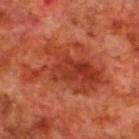workup: total-body-photography surveillance lesion; no biopsy | TBP lesion metrics: a nevus-likeness score of about 20/100 and a lesion-detection confidence of about 100/100 | size: ≈9.5 mm | image: ~15 mm crop, total-body skin-cancer survey | tile lighting: cross-polarized illumination | subject: male, aged 68 to 72 | body site: the upper back.